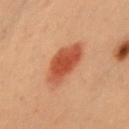Notes:
* workup — imaged on a skin check; not biopsied
* patient — female, aged approximately 40
* anatomic site — the front of the torso
* image source — ~15 mm crop, total-body skin-cancer survey
* lesion diameter — about 6.5 mm
* lighting — cross-polarized illumination